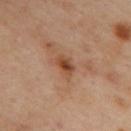Captured during whole-body skin photography for melanoma surveillance; the lesion was not biopsied.
From the upper back.
The tile uses cross-polarized illumination.
Approximately 3 mm at its widest.
A female patient, about 40 years old.
A region of skin cropped from a whole-body photographic capture, roughly 15 mm wide.